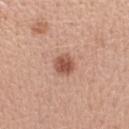Captured during whole-body skin photography for melanoma surveillance; the lesion was not biopsied.
An algorithmic analysis of the crop reported a lesion area of about 5.5 mm² and a symmetry-axis asymmetry near 0.2. It also reported a lesion color around L≈54 a*≈23 b*≈30 in CIELAB, about 13 CIELAB-L* units darker than the surrounding skin, and a normalized lesion–skin contrast near 8.5.
On the right upper arm.
The tile uses white-light illumination.
About 3 mm across.
A lesion tile, about 15 mm wide, cut from a 3D total-body photograph.
The patient is a female aged approximately 40.